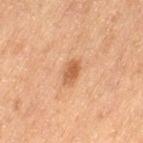Impression: Part of a total-body skin-imaging series; this lesion was reviewed on a skin check and was not flagged for biopsy. Context: From the left thigh. A roughly 15 mm field-of-view crop from a total-body skin photograph. This is a cross-polarized tile. The subject is a female about 55 years old. Automated image analysis of the tile measured an outline eccentricity of about 0.8 (0 = round, 1 = elongated) and a shape-asymmetry score of about 0.2 (0 = symmetric). It also reported a mean CIELAB color near L≈51 a*≈21 b*≈35, roughly 10 lightness units darker than nearby skin, and a normalized border contrast of about 7.5. And it measured border irregularity of about 2 on a 0–10 scale, a color-variation rating of about 2/10, and peripheral color asymmetry of about 0.5.